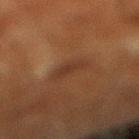Recorded during total-body skin imaging; not selected for excision or biopsy. Located on the right lower leg. Automated tile analysis of the lesion measured an area of roughly 6 mm² and a shape eccentricity near 0.85. The analysis additionally found an average lesion color of about L≈28 a*≈17 b*≈25 (CIELAB) and a normalized lesion–skin contrast near 6. Imaged with cross-polarized lighting. About 3.5 mm across. This image is a 15 mm lesion crop taken from a total-body photograph. The patient is a male about 65 years old.